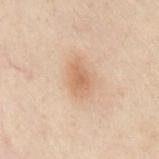Impression: Recorded during total-body skin imaging; not selected for excision or biopsy. Acquisition and patient details: A male patient aged around 50. Cropped from a whole-body photographic skin survey; the tile spans about 15 mm. The lesion is on the chest.